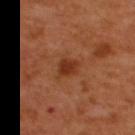Q: Lesion location?
A: the upper back
Q: How was this image acquired?
A: 15 mm crop, total-body photography
Q: What did automated image analysis measure?
A: an area of roughly 5 mm², an outline eccentricity of about 0.7 (0 = round, 1 = elongated), and a shape-asymmetry score of about 0.2 (0 = symmetric); a border-irregularity rating of about 1.5/10 and radial color variation of about 0.5
Q: Lesion size?
A: ~2.5 mm (longest diameter)
Q: How was the tile lit?
A: cross-polarized
Q: Patient demographics?
A: male, aged approximately 50